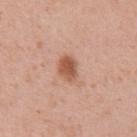The tile uses white-light illumination.
A male subject, roughly 55 years of age.
Longest diameter approximately 3 mm.
On the front of the torso.
A region of skin cropped from a whole-body photographic capture, roughly 15 mm wide.